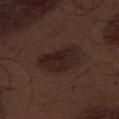Recorded during total-body skin imaging; not selected for excision or biopsy. A male patient, aged 68–72. The lesion's longest dimension is about 5 mm. On the front of the torso. The tile uses white-light illumination. Automated image analysis of the tile measured an area of roughly 13 mm², an eccentricity of roughly 0.8, and a symmetry-axis asymmetry near 0.3. The analysis additionally found an average lesion color of about L≈21 a*≈16 b*≈17 (CIELAB). The analysis additionally found a color-variation rating of about 3/10 and a peripheral color-asymmetry measure near 1. The software also gave a nevus-likeness score of about 75/100. This image is a 15 mm lesion crop taken from a total-body photograph.